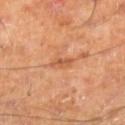Captured during whole-body skin photography for melanoma surveillance; the lesion was not biopsied. Automated tile analysis of the lesion measured a shape eccentricity near 0.85 and two-axis asymmetry of about 0.3. The software also gave an automated nevus-likeness rating near 0 out of 100. The tile uses cross-polarized illumination. The lesion's longest dimension is about 2.5 mm. A 15 mm crop from a total-body photograph taken for skin-cancer surveillance. The lesion is on the right lower leg. The subject is a male approximately 70 years of age.